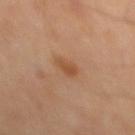Clinical impression:
Recorded during total-body skin imaging; not selected for excision or biopsy.
Clinical summary:
Located on the mid back. A male patient aged 68 to 72. A region of skin cropped from a whole-body photographic capture, roughly 15 mm wide. The recorded lesion diameter is about 2.5 mm. Imaged with cross-polarized lighting.